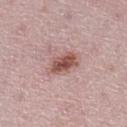  biopsy_status: not biopsied; imaged during a skin examination
  site: left lower leg
  lighting: white-light
  patient:
    sex: female
    age_approx: 45
  image:
    source: total-body photography crop
    field_of_view_mm: 15
  automated_metrics:
    area_mm2_approx: 7.5
    eccentricity: 0.75
    nevus_likeness_0_100: 80
    lesion_detection_confidence_0_100: 100